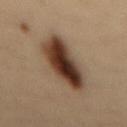biopsy_status: not biopsied; imaged during a skin examination
image:
  source: total-body photography crop
  field_of_view_mm: 15
lighting: cross-polarized
automated_metrics:
  cielab_L: 34
  cielab_a: 15
  cielab_b: 25
  vs_skin_darker_L: 17.0
  vs_skin_contrast_norm: 14.0
  nevus_likeness_0_100: 100
  lesion_detection_confidence_0_100: 100
patient:
  sex: male
  age_approx: 35
site: lower back
lesion_size:
  long_diameter_mm_approx: 8.0